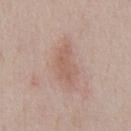Assessment: The lesion was photographed on a routine skin check and not biopsied; there is no pathology result. Context: The recorded lesion diameter is about 4.5 mm. From the chest. A lesion tile, about 15 mm wide, cut from a 3D total-body photograph. This is a white-light tile. The total-body-photography lesion software estimated a within-lesion color-variation index near 2/10 and radial color variation of about 1. The analysis additionally found lesion-presence confidence of about 100/100. A male patient, about 40 years old.